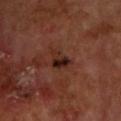Part of a total-body skin-imaging series; this lesion was reviewed on a skin check and was not flagged for biopsy. The lesion is located on the head or neck. The lesion's longest dimension is about 3.5 mm. Cropped from a total-body skin-imaging series; the visible field is about 15 mm. A male subject aged 68–72. Captured under cross-polarized illumination.